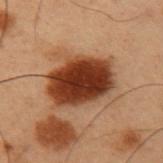<record>
  <biopsy_status>not biopsied; imaged during a skin examination</biopsy_status>
  <site>left upper arm</site>
  <lighting>cross-polarized</lighting>
  <image>
    <source>total-body photography crop</source>
    <field_of_view_mm>15</field_of_view_mm>
  </image>
  <lesion_size>
    <long_diameter_mm_approx>6.5</long_diameter_mm_approx>
  </lesion_size>
  <automated_metrics>
    <cielab_L>30</cielab_L>
    <cielab_a>22</cielab_a>
    <cielab_b>28</cielab_b>
    <vs_skin_darker_L>17.0</vs_skin_darker_L>
    <vs_skin_contrast_norm>15.0</vs_skin_contrast_norm>
  </automated_metrics>
  <patient>
    <sex>male</sex>
    <age_approx>55</age_approx>
  </patient>
</record>